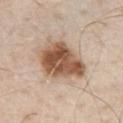Imaged during a routine full-body skin examination; the lesion was not biopsied and no histopathology is available. The recorded lesion diameter is about 6 mm. A male subject, aged around 80. A 15 mm crop from a total-body photograph taken for skin-cancer surveillance. The lesion is located on the chest.